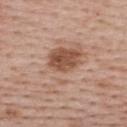notes: imaged on a skin check; not biopsied
subject: female, aged 53 to 57
size: ~7 mm (longest diameter)
location: the upper back
imaging modality: total-body-photography crop, ~15 mm field of view
tile lighting: white-light
TBP lesion metrics: a lesion area of about 22 mm² and a shape-asymmetry score of about 0.4 (0 = symmetric)